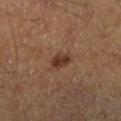No biopsy was performed on this lesion — it was imaged during a full skin examination and was not determined to be concerning. Automated image analysis of the tile measured a lesion area of about 4.5 mm² and a symmetry-axis asymmetry near 0.2. And it measured an average lesion color of about L≈32 a*≈18 b*≈25 (CIELAB) and a normalized border contrast of about 8.5. The software also gave a border-irregularity index near 2/10, internal color variation of about 2.5 on a 0–10 scale, and radial color variation of about 1. A male subject, aged approximately 60. From the right lower leg. Imaged with cross-polarized lighting. Measured at roughly 2.5 mm in maximum diameter. A lesion tile, about 15 mm wide, cut from a 3D total-body photograph.